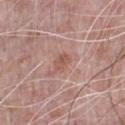The lesion was photographed on a routine skin check and not biopsied; there is no pathology result.
An algorithmic analysis of the crop reported a footprint of about 3.5 mm², a shape eccentricity near 0.8, and two-axis asymmetry of about 0.3. The analysis additionally found an automated nevus-likeness rating near 0 out of 100 and lesion-presence confidence of about 100/100.
This image is a 15 mm lesion crop taken from a total-body photograph.
Captured under white-light illumination.
A male patient roughly 70 years of age.
Longest diameter approximately 2.5 mm.
On the chest.